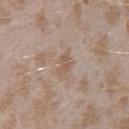Recorded during total-body skin imaging; not selected for excision or biopsy.
The lesion's longest dimension is about 3 mm.
A lesion tile, about 15 mm wide, cut from a 3D total-body photograph.
This is a white-light tile.
A female subject, in their mid- to late 20s.
The lesion-visualizer software estimated a mean CIELAB color near L≈56 a*≈15 b*≈28, roughly 7 lightness units darker than nearby skin, and a normalized border contrast of about 5.5. The software also gave a border-irregularity index near 4/10 and radial color variation of about 0.5.
From the arm.